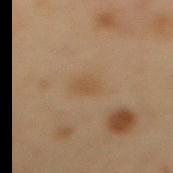Cropped from a whole-body photographic skin survey; the tile spans about 15 mm. This is a cross-polarized tile. The lesion is on the mid back. A male subject aged 53–57.